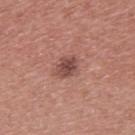Impression: This lesion was catalogued during total-body skin photography and was not selected for biopsy. Context: Captured under white-light illumination. From the upper back. A 15 mm close-up extracted from a 3D total-body photography capture. Longest diameter approximately 3 mm. The patient is a male aged around 40.